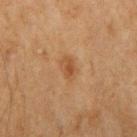Imaged during a routine full-body skin examination; the lesion was not biopsied and no histopathology is available.
Approximately 2.5 mm at its widest.
A male patient, aged approximately 50.
The lesion is located on the right upper arm.
A lesion tile, about 15 mm wide, cut from a 3D total-body photograph.